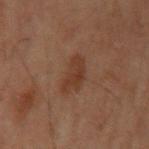Background: This is a cross-polarized tile. A male subject aged approximately 60. A 15 mm crop from a total-body photograph taken for skin-cancer surveillance. The lesion is on the left upper arm.